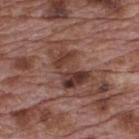notes — total-body-photography surveillance lesion; no biopsy
image source — 15 mm crop, total-body photography
subject — male, aged 68 to 72
location — the upper back
lighting — white-light illumination
TBP lesion metrics — an outline eccentricity of about 0.8 (0 = round, 1 = elongated); internal color variation of about 8 on a 0–10 scale; lesion-presence confidence of about 100/100